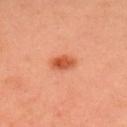image-analysis metrics: a lesion–skin lightness drop of about 10 and a lesion-to-skin contrast of about 8.5 (normalized; higher = more distinct)
body site: the head or neck
subject: female, aged approximately 40
imaging modality: total-body-photography crop, ~15 mm field of view
lesion diameter: about 2.5 mm